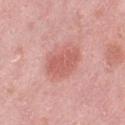biopsy status: imaged on a skin check; not biopsied
automated metrics: a mean CIELAB color near L≈60 a*≈29 b*≈26, a lesion–skin lightness drop of about 9, and a normalized border contrast of about 6; a border-irregularity index near 2/10, a within-lesion color-variation index near 2/10, and a peripheral color-asymmetry measure near 0.5
illumination: white-light
lesion size: ≈4.5 mm
location: the leg
image source: ~15 mm crop, total-body skin-cancer survey
subject: female, in their 40s